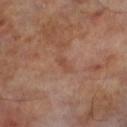No biopsy was performed on this lesion — it was imaged during a full skin examination and was not determined to be concerning.
Longest diameter approximately 2.5 mm.
The subject is a male approximately 70 years of age.
Imaged with cross-polarized lighting.
From the left lower leg.
This image is a 15 mm lesion crop taken from a total-body photograph.